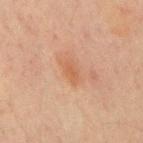The lesion was photographed on a routine skin check and not biopsied; there is no pathology result. Cropped from a whole-body photographic skin survey; the tile spans about 15 mm. About 3 mm across. An algorithmic analysis of the crop reported a footprint of about 3 mm². The software also gave a lesion color around L≈48 a*≈20 b*≈30 in CIELAB and a normalized lesion–skin contrast near 5.5. It also reported a border-irregularity index near 2.5/10, a within-lesion color-variation index near 1/10, and peripheral color asymmetry of about 0.5. On the chest. A male subject approximately 70 years of age. This is a cross-polarized tile.